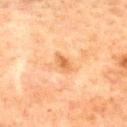Image and clinical context: A female patient aged approximately 60. From the upper back. The total-body-photography lesion software estimated an area of roughly 3 mm² and an outline eccentricity of about 0.8 (0 = round, 1 = elongated). The software also gave border irregularity of about 3 on a 0–10 scale and radial color variation of about 0.5. It also reported a nevus-likeness score of about 0/100 and lesion-presence confidence of about 100/100. The lesion's longest dimension is about 2.5 mm. The tile uses cross-polarized illumination. A 15 mm close-up extracted from a 3D total-body photography capture.